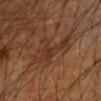- workup — catalogued during a skin exam; not biopsied
- automated lesion analysis — a lesion area of about 14 mm², an eccentricity of roughly 0.85, and a symmetry-axis asymmetry near 0.55
- subject — male, aged around 60
- acquisition — 15 mm crop, total-body photography
- location — the left forearm
- diameter — ≈6.5 mm